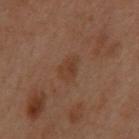The patient is a female aged 53 to 57.
Cropped from a whole-body photographic skin survey; the tile spans about 15 mm.
The lesion is located on the upper back.
Imaged with cross-polarized lighting.